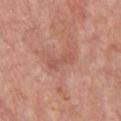This lesion was catalogued during total-body skin photography and was not selected for biopsy.
About 3.5 mm across.
Imaged with white-light lighting.
Cropped from a whole-body photographic skin survey; the tile spans about 15 mm.
A male subject aged around 60.
The lesion is located on the chest.